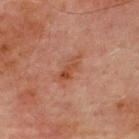Q: What is the imaging modality?
A: ~15 mm tile from a whole-body skin photo
Q: What is the anatomic site?
A: the chest
Q: What are the patient's age and sex?
A: male, about 70 years old